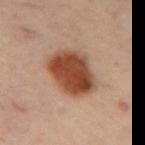follow-up = total-body-photography surveillance lesion; no biopsy
lighting = cross-polarized illumination
patient = male, in their 50s
anatomic site = the back
lesion diameter = ~5.5 mm (longest diameter)
acquisition = 15 mm crop, total-body photography